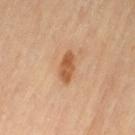biopsy status: no biopsy performed (imaged during a skin exam) | body site: the left thigh | illumination: cross-polarized illumination | automated metrics: a footprint of about 6 mm², an outline eccentricity of about 0.85 (0 = round, 1 = elongated), and two-axis asymmetry of about 0.15; a mean CIELAB color near L≈58 a*≈24 b*≈39, roughly 12 lightness units darker than nearby skin, and a lesion-to-skin contrast of about 8 (normalized; higher = more distinct); internal color variation of about 3 on a 0–10 scale and radial color variation of about 1; a classifier nevus-likeness of about 90/100 and a detector confidence of about 100 out of 100 that the crop contains a lesion | subject: female, about 70 years old | acquisition: ~15 mm tile from a whole-body skin photo.